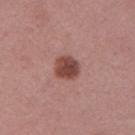follow-up: total-body-photography surveillance lesion; no biopsy | image: total-body-photography crop, ~15 mm field of view | lighting: white-light illumination | subject: female, aged 48 to 52 | automated lesion analysis: a lesion area of about 6 mm² and an outline eccentricity of about 0.45 (0 = round, 1 = elongated); an average lesion color of about L≈45 a*≈24 b*≈24 (CIELAB), a lesion–skin lightness drop of about 14, and a normalized border contrast of about 10.5; border irregularity of about 2 on a 0–10 scale, a color-variation rating of about 3/10, and peripheral color asymmetry of about 1 | lesion diameter: ≈2.5 mm | anatomic site: the right upper arm.